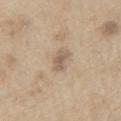Impression:
This lesion was catalogued during total-body skin photography and was not selected for biopsy.
Background:
From the arm. A male subject roughly 70 years of age. The lesion's longest dimension is about 3 mm. This is a white-light tile. Cropped from a whole-body photographic skin survey; the tile spans about 15 mm.